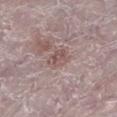workup=imaged on a skin check; not biopsied
subject=female, aged 63 to 67
image source=15 mm crop, total-body photography
location=the leg